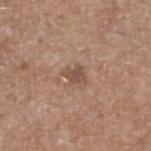biopsy status — imaged on a skin check; not biopsied | site — the right lower leg | size — ≈2.5 mm | patient — male, roughly 65 years of age | lighting — white-light | imaging modality — ~15 mm tile from a whole-body skin photo.